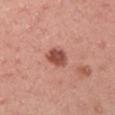No biopsy was performed on this lesion — it was imaged during a full skin examination and was not determined to be concerning. The patient is a male roughly 40 years of age. A roughly 15 mm field-of-view crop from a total-body skin photograph. The total-body-photography lesion software estimated an area of roughly 6 mm², a shape eccentricity near 0.6, and a shape-asymmetry score of about 0.2 (0 = symmetric). It also reported an average lesion color of about L≈51 a*≈28 b*≈28 (CIELAB) and a normalized border contrast of about 9.5. The software also gave internal color variation of about 2.5 on a 0–10 scale and a peripheral color-asymmetry measure near 1. The analysis additionally found lesion-presence confidence of about 100/100. On the left upper arm.